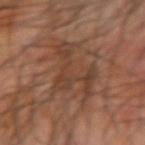The lesion was photographed on a routine skin check and not biopsied; there is no pathology result.
A roughly 15 mm field-of-view crop from a total-body skin photograph.
A male subject aged around 65.
The lesion-visualizer software estimated an automated nevus-likeness rating near 0 out of 100 and a detector confidence of about 95 out of 100 that the crop contains a lesion.
From the arm.